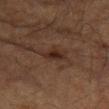No biopsy was performed on this lesion — it was imaged during a full skin examination and was not determined to be concerning. This image is a 15 mm lesion crop taken from a total-body photograph. Imaged with cross-polarized lighting. The subject is a male aged approximately 85 or older. The lesion is located on the left forearm. The lesion-visualizer software estimated an area of roughly 5 mm², a shape eccentricity near 0.8, and a symmetry-axis asymmetry near 0.2. The analysis additionally found an average lesion color of about L≈23 a*≈15 b*≈21 (CIELAB), about 7 CIELAB-L* units darker than the surrounding skin, and a lesion-to-skin contrast of about 7.5 (normalized; higher = more distinct). The software also gave border irregularity of about 2.5 on a 0–10 scale, internal color variation of about 2.5 on a 0–10 scale, and radial color variation of about 1. It also reported a nevus-likeness score of about 70/100.